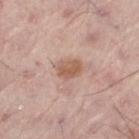<record>
<biopsy_status>not biopsied; imaged during a skin examination</biopsy_status>
<lighting>white-light</lighting>
<image>
  <source>total-body photography crop</source>
  <field_of_view_mm>15</field_of_view_mm>
</image>
<automated_metrics>
  <cielab_L>58</cielab_L>
  <cielab_a>20</cielab_a>
  <cielab_b>29</cielab_b>
  <vs_skin_darker_L>10.0</vs_skin_darker_L>
  <vs_skin_contrast_norm>7.5</vs_skin_contrast_norm>
  <border_irregularity_0_10>1.5</border_irregularity_0_10>
  <color_variation_0_10>3.0</color_variation_0_10>
  <nevus_likeness_0_100>35</nevus_likeness_0_100>
</automated_metrics>
<site>left thigh</site>
<patient>
  <sex>male</sex>
  <age_approx>55</age_approx>
</patient>
</record>